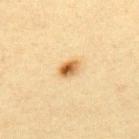Notes:
* biopsy status — catalogued during a skin exam; not biopsied
* tile lighting — cross-polarized illumination
* site — the upper back
* image source — ~15 mm tile from a whole-body skin photo
* subject — female, about 40 years old
* automated lesion analysis — a mean CIELAB color near L≈54 a*≈18 b*≈39, a lesion–skin lightness drop of about 15, and a lesion-to-skin contrast of about 10.5 (normalized; higher = more distinct); a border-irregularity rating of about 1/10, a within-lesion color-variation index near 8.5/10, and peripheral color asymmetry of about 3.5; a nevus-likeness score of about 100/100 and a detector confidence of about 100 out of 100 that the crop contains a lesion
* lesion size — ≈2.5 mm